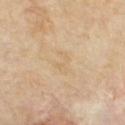Recorded during total-body skin imaging; not selected for excision or biopsy. Longest diameter approximately 2.5 mm. Cropped from a total-body skin-imaging series; the visible field is about 15 mm. On the chest. The subject is a male aged around 70.